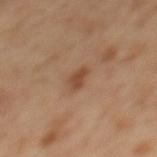The lesion was photographed on a routine skin check and not biopsied; there is no pathology result. Longest diameter approximately 2.5 mm. Located on the back. A male patient, roughly 60 years of age. A 15 mm close-up extracted from a 3D total-body photography capture.